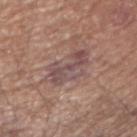biopsy_status: not biopsied; imaged during a skin examination
site: right upper arm
patient:
  sex: male
  age_approx: 65
lesion_size:
  long_diameter_mm_approx: 5.5
image:
  source: total-body photography crop
  field_of_view_mm: 15
lighting: white-light